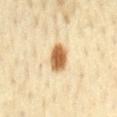The lesion was tiled from a total-body skin photograph and was not biopsied. Captured under cross-polarized illumination. Longest diameter approximately 3.5 mm. From the mid back. Cropped from a total-body skin-imaging series; the visible field is about 15 mm. A female subject, roughly 35 years of age.